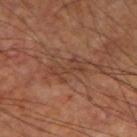Clinical summary:
Automated tile analysis of the lesion measured an outline eccentricity of about 0.9 (0 = round, 1 = elongated). And it measured border irregularity of about 8 on a 0–10 scale and a peripheral color-asymmetry measure near 0.5. It also reported a classifier nevus-likeness of about 0/100 and lesion-presence confidence of about 80/100. The lesion's longest dimension is about 4.5 mm. Located on the left thigh. Captured under cross-polarized illumination. A roughly 15 mm field-of-view crop from a total-body skin photograph. The patient is a male in their 60s.